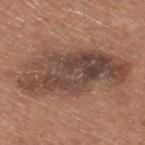workup: catalogued during a skin exam; not biopsied | lesion size: ~12 mm (longest diameter) | patient: male, in their mid- to late 60s | anatomic site: the upper back | imaging modality: total-body-photography crop, ~15 mm field of view | tile lighting: white-light illumination | image-analysis metrics: a footprint of about 45 mm², an outline eccentricity of about 0.9 (0 = round, 1 = elongated), and a symmetry-axis asymmetry near 0.25; a mean CIELAB color near L≈45 a*≈18 b*≈25 and a normalized border contrast of about 9.5.